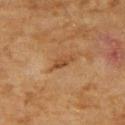This lesion was catalogued during total-body skin photography and was not selected for biopsy. A female subject aged 58 to 62. An algorithmic analysis of the crop reported about 8 CIELAB-L* units darker than the surrounding skin. The analysis additionally found a border-irregularity index near 2.5/10 and a within-lesion color-variation index near 0.5/10. A 15 mm crop from a total-body photograph taken for skin-cancer surveillance. The tile uses cross-polarized illumination. On the right upper arm. The lesion's longest dimension is about 2.5 mm.